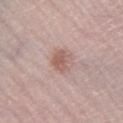Q: Was this lesion biopsied?
A: imaged on a skin check; not biopsied
Q: What kind of image is this?
A: 15 mm crop, total-body photography
Q: Who is the patient?
A: female, approximately 65 years of age
Q: Where on the body is the lesion?
A: the right lower leg
Q: What did automated image analysis measure?
A: a footprint of about 6 mm² and an eccentricity of roughly 0.5; a mean CIELAB color near L≈58 a*≈20 b*≈24, about 9 CIELAB-L* units darker than the surrounding skin, and a normalized border contrast of about 6.5; border irregularity of about 2.5 on a 0–10 scale and a within-lesion color-variation index near 2.5/10; a classifier nevus-likeness of about 75/100 and a lesion-detection confidence of about 100/100